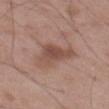An algorithmic analysis of the crop reported an eccentricity of roughly 0.85 and two-axis asymmetry of about 0.35. And it measured a mean CIELAB color near L≈49 a*≈19 b*≈25, a lesion–skin lightness drop of about 9, and a normalized border contrast of about 7. And it measured border irregularity of about 4 on a 0–10 scale, a within-lesion color-variation index near 3.5/10, and peripheral color asymmetry of about 1. A close-up tile cropped from a whole-body skin photograph, about 15 mm across. The lesion is located on the left thigh. The recorded lesion diameter is about 5 mm. A male subject aged 48 to 52. This is a white-light tile.